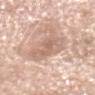Captured during whole-body skin photography for melanoma surveillance; the lesion was not biopsied. A female patient about 75 years old. The lesion is on the head or neck. A 15 mm close-up tile from a total-body photography series done for melanoma screening.